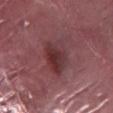biopsy status — no biopsy performed (imaged during a skin exam)
subject — male, aged around 40
lighting — white-light
location — the left lower leg
lesion size — ≈4.5 mm
imaging modality — total-body-photography crop, ~15 mm field of view